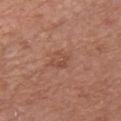This lesion was catalogued during total-body skin photography and was not selected for biopsy. A 15 mm crop from a total-body photograph taken for skin-cancer surveillance. On the chest. The recorded lesion diameter is about 3 mm. A male subject aged approximately 75. An algorithmic analysis of the crop reported a normalized lesion–skin contrast near 5. It also reported a classifier nevus-likeness of about 0/100 and a lesion-detection confidence of about 100/100. This is a white-light tile.